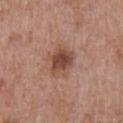Recorded during total-body skin imaging; not selected for excision or biopsy. About 3.5 mm across. A region of skin cropped from a whole-body photographic capture, roughly 15 mm wide. The patient is a male about 70 years old. The lesion-visualizer software estimated a shape eccentricity near 0.45 and two-axis asymmetry of about 0.2. And it measured an average lesion color of about L≈47 a*≈22 b*≈27 (CIELAB), a lesion–skin lightness drop of about 12, and a normalized border contrast of about 8.5. The analysis additionally found border irregularity of about 2.5 on a 0–10 scale and internal color variation of about 4 on a 0–10 scale. On the chest.